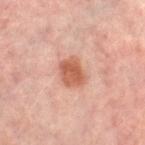This lesion was catalogued during total-body skin photography and was not selected for biopsy. On the left thigh. A female subject, aged around 50. Approximately 3.5 mm at its widest. The tile uses cross-polarized illumination. A 15 mm crop from a total-body photograph taken for skin-cancer surveillance. An algorithmic analysis of the crop reported a mean CIELAB color near L≈57 a*≈26 b*≈31 and a normalized lesion–skin contrast near 8.5. It also reported a border-irregularity index near 1.5/10, a within-lesion color-variation index near 3.5/10, and peripheral color asymmetry of about 1.5. It also reported a nevus-likeness score of about 95/100 and a lesion-detection confidence of about 100/100.